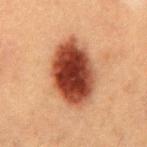This lesion was catalogued during total-body skin photography and was not selected for biopsy. A 15 mm close-up extracted from a 3D total-body photography capture. The tile uses cross-polarized illumination. A male patient, in their 50s. On the mid back. The recorded lesion diameter is about 7.5 mm. The total-body-photography lesion software estimated a footprint of about 26 mm², an outline eccentricity of about 0.8 (0 = round, 1 = elongated), and a symmetry-axis asymmetry near 0.1. And it measured an average lesion color of about L≈36 a*≈25 b*≈28 (CIELAB), roughly 20 lightness units darker than nearby skin, and a lesion-to-skin contrast of about 15.5 (normalized; higher = more distinct). And it measured internal color variation of about 7 on a 0–10 scale and a peripheral color-asymmetry measure near 2. And it measured a classifier nevus-likeness of about 100/100 and a detector confidence of about 100 out of 100 that the crop contains a lesion.